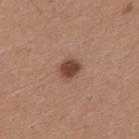biopsy status: imaged on a skin check; not biopsied | body site: the back | acquisition: total-body-photography crop, ~15 mm field of view | subject: male, aged around 30 | automated lesion analysis: an area of roughly 5.5 mm², an eccentricity of roughly 0.5, and a symmetry-axis asymmetry near 0.25; a mean CIELAB color near L≈45 a*≈20 b*≈27, a lesion–skin lightness drop of about 13, and a normalized lesion–skin contrast near 9.5; a border-irregularity index near 2/10 and radial color variation of about 1; an automated nevus-likeness rating near 95 out of 100 and a detector confidence of about 100 out of 100 that the crop contains a lesion.